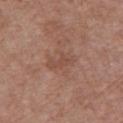{
  "biopsy_status": "not biopsied; imaged during a skin examination",
  "lighting": "white-light",
  "site": "chest",
  "image": {
    "source": "total-body photography crop",
    "field_of_view_mm": 15
  },
  "patient": {
    "sex": "female",
    "age_approx": 75
  }
}